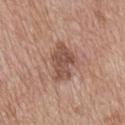<case>
<lesion_size>
  <long_diameter_mm_approx>5.0</long_diameter_mm_approx>
</lesion_size>
<site>mid back</site>
<image>
  <source>total-body photography crop</source>
  <field_of_view_mm>15</field_of_view_mm>
</image>
<patient>
  <sex>male</sex>
  <age_approx>60</age_approx>
</patient>
<lighting>white-light</lighting>
<automated_metrics>
  <area_mm2_approx>11.0</area_mm2_approx>
  <eccentricity>0.8</eccentricity>
  <shape_asymmetry>0.3</shape_asymmetry>
  <cielab_L>51</cielab_L>
  <cielab_a>20</cielab_a>
  <cielab_b>26</cielab_b>
  <vs_skin_contrast_norm>7.5</vs_skin_contrast_norm>
  <border_irregularity_0_10>3.5</border_irregularity_0_10>
  <color_variation_0_10>3.5</color_variation_0_10>
  <peripheral_color_asymmetry>1.0</peripheral_color_asymmetry>
</automated_metrics>
</case>